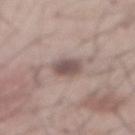Impression: This lesion was catalogued during total-body skin photography and was not selected for biopsy. Acquisition and patient details: An algorithmic analysis of the crop reported a lesion area of about 6 mm² and a symmetry-axis asymmetry near 0.2. It also reported border irregularity of about 1.5 on a 0–10 scale, a color-variation rating of about 2.5/10, and radial color variation of about 1. The software also gave a classifier nevus-likeness of about 60/100 and lesion-presence confidence of about 100/100. A region of skin cropped from a whole-body photographic capture, roughly 15 mm wide. A male subject, aged approximately 55. From the abdomen. The lesion's longest dimension is about 3 mm.